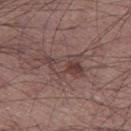<case>
  <biopsy_status>not biopsied; imaged during a skin examination</biopsy_status>
  <site>left thigh</site>
  <image>
    <source>total-body photography crop</source>
    <field_of_view_mm>15</field_of_view_mm>
  </image>
  <patient>
    <sex>male</sex>
    <age_approx>55</age_approx>
  </patient>
</case>